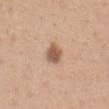Impression:
This lesion was catalogued during total-body skin photography and was not selected for biopsy.
Acquisition and patient details:
Located on the chest. This is a white-light tile. Automated tile analysis of the lesion measured a footprint of about 4.5 mm², an outline eccentricity of about 0.6 (0 = round, 1 = elongated), and two-axis asymmetry of about 0.2. The analysis additionally found about 14 CIELAB-L* units darker than the surrounding skin. It also reported border irregularity of about 1.5 on a 0–10 scale, a color-variation rating of about 2.5/10, and peripheral color asymmetry of about 1. A 15 mm close-up extracted from a 3D total-body photography capture. A female patient, aged around 30.